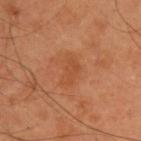notes = imaged on a skin check; not biopsied
image source = 15 mm crop, total-body photography
body site = the back
lesion size = ≈3.5 mm
illumination = cross-polarized
patient = male, about 55 years old